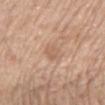follow-up: imaged on a skin check; not biopsied
patient: male, aged approximately 70
image: ~15 mm tile from a whole-body skin photo
size: about 2.5 mm
anatomic site: the back